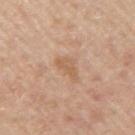The lesion was tiled from a total-body skin photograph and was not biopsied. Longest diameter approximately 3.5 mm. A male patient, approximately 55 years of age. A roughly 15 mm field-of-view crop from a total-body skin photograph. Captured under white-light illumination. On the right upper arm.